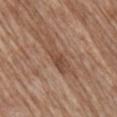Imaged during a routine full-body skin examination; the lesion was not biopsied and no histopathology is available. A 15 mm crop from a total-body photograph taken for skin-cancer surveillance. This is a white-light tile. A male patient, in their 70s. Longest diameter approximately 6 mm. Located on the right upper arm. An algorithmic analysis of the crop reported a border-irregularity rating of about 6/10 and radial color variation of about 1.